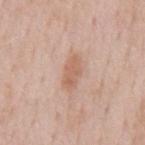The tile uses white-light illumination.
Cropped from a whole-body photographic skin survey; the tile spans about 15 mm.
A male subject in their 60s.
Located on the mid back.
About 4 mm across.